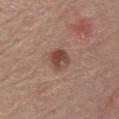The lesion was tiled from a total-body skin photograph and was not biopsied. A male patient roughly 70 years of age. The lesion-visualizer software estimated a footprint of about 6.5 mm², an outline eccentricity of about 0.35 (0 = round, 1 = elongated), and a symmetry-axis asymmetry near 0.15. It also reported a mean CIELAB color near L≈46 a*≈21 b*≈26 and a normalized lesion–skin contrast near 8.5. And it measured a border-irregularity index near 1.5/10 and radial color variation of about 1.5. Approximately 2.5 mm at its widest. The lesion is located on the chest. Imaged with white-light lighting. A 15 mm close-up extracted from a 3D total-body photography capture.